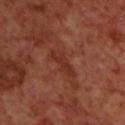| field | value |
|---|---|
| workup | imaged on a skin check; not biopsied |
| site | the upper back |
| illumination | cross-polarized |
| TBP lesion metrics | a lesion color around L≈31 a*≈26 b*≈28 in CIELAB, roughly 6 lightness units darker than nearby skin, and a normalized border contrast of about 6; an automated nevus-likeness rating near 0 out of 100 and a detector confidence of about 50 out of 100 that the crop contains a lesion |
| patient | male, aged around 70 |
| image source | 15 mm crop, total-body photography |
| lesion size | ≈4 mm |